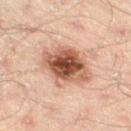workup = catalogued during a skin exam; not biopsied